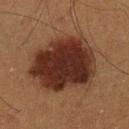Captured during whole-body skin photography for melanoma surveillance; the lesion was not biopsied.
The lesion-visualizer software estimated a shape eccentricity near 0.6. The analysis additionally found an average lesion color of about L≈24 a*≈18 b*≈22 (CIELAB) and a normalized lesion–skin contrast near 14. The software also gave a detector confidence of about 100 out of 100 that the crop contains a lesion.
Captured under cross-polarized illumination.
The lesion is located on the left lower leg.
A male patient, about 40 years old.
A roughly 15 mm field-of-view crop from a total-body skin photograph.